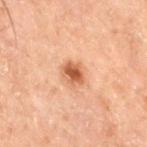follow-up=no biopsy performed (imaged during a skin exam); acquisition=~15 mm tile from a whole-body skin photo; site=the right thigh; patient=male, about 70 years old; image-analysis metrics=a classifier nevus-likeness of about 90/100 and a detector confidence of about 100 out of 100 that the crop contains a lesion.